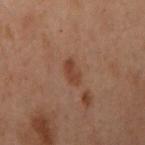This lesion was catalogued during total-body skin photography and was not selected for biopsy. The lesion is on the left arm. The tile uses cross-polarized illumination. An algorithmic analysis of the crop reported an area of roughly 4.5 mm², an eccentricity of roughly 0.8, and two-axis asymmetry of about 0.15. The analysis additionally found a border-irregularity rating of about 1.5/10 and a peripheral color-asymmetry measure near 1. The analysis additionally found a lesion-detection confidence of about 100/100. The lesion's longest dimension is about 3 mm. A female subject in their mid-50s. A lesion tile, about 15 mm wide, cut from a 3D total-body photograph.